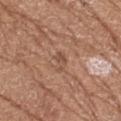{"image": {"source": "total-body photography crop", "field_of_view_mm": 15}, "lesion_size": {"long_diameter_mm_approx": 2.5}, "lighting": "white-light", "site": "left upper arm", "automated_metrics": {"border_irregularity_0_10": 4.5, "nevus_likeness_0_100": 0, "lesion_detection_confidence_0_100": 100}, "patient": {"sex": "female", "age_approx": 75}}